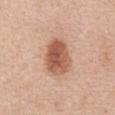Q: Was a biopsy performed?
A: no biopsy performed (imaged during a skin exam)
Q: How large is the lesion?
A: ≈4.5 mm
Q: Who is the patient?
A: male, aged around 70
Q: Lesion location?
A: the abdomen
Q: What kind of image is this?
A: total-body-photography crop, ~15 mm field of view